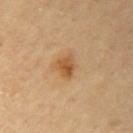Recorded during total-body skin imaging; not selected for excision or biopsy. Cropped from a whole-body photographic skin survey; the tile spans about 15 mm. From the right upper arm. A male patient, aged around 85 or older. The tile uses cross-polarized illumination.